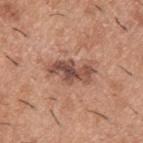The lesion was tiled from a total-body skin photograph and was not biopsied. The lesion is on the upper back. Captured under white-light illumination. A male patient, aged 38 to 42. The total-body-photography lesion software estimated a lesion area of about 11 mm² and an eccentricity of roughly 0.9. The software also gave a border-irregularity index near 4/10 and a peripheral color-asymmetry measure near 2. And it measured an automated nevus-likeness rating near 35 out of 100 and a detector confidence of about 100 out of 100 that the crop contains a lesion. A 15 mm close-up extracted from a 3D total-body photography capture. Measured at roughly 5.5 mm in maximum diameter.